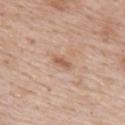Automated tile analysis of the lesion measured an outline eccentricity of about 0.9 (0 = round, 1 = elongated) and two-axis asymmetry of about 0.2. And it measured a border-irregularity index near 2.5/10 and a peripheral color-asymmetry measure near 0.
A 15 mm close-up tile from a total-body photography series done for melanoma screening.
Located on the upper back.
The subject is a female roughly 70 years of age.
Imaged with white-light lighting.
Longest diameter approximately 2.5 mm.